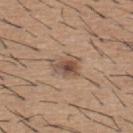No biopsy was performed on this lesion — it was imaged during a full skin examination and was not determined to be concerning.
From the upper back.
Automated image analysis of the tile measured a footprint of about 7 mm², an outline eccentricity of about 0.75 (0 = round, 1 = elongated), and two-axis asymmetry of about 0.3. The analysis additionally found a classifier nevus-likeness of about 85/100 and a detector confidence of about 100 out of 100 that the crop contains a lesion.
Imaged with white-light lighting.
About 3.5 mm across.
Cropped from a whole-body photographic skin survey; the tile spans about 15 mm.
A male subject, aged 58–62.